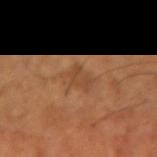This lesion was catalogued during total-body skin photography and was not selected for biopsy.
A 15 mm crop from a total-body photograph taken for skin-cancer surveillance.
The subject is a male about 50 years old.
Approximately 3.5 mm at its widest.
Automated tile analysis of the lesion measured a mean CIELAB color near L≈46 a*≈21 b*≈34, a lesion–skin lightness drop of about 6, and a lesion-to-skin contrast of about 5 (normalized; higher = more distinct). It also reported border irregularity of about 4 on a 0–10 scale and peripheral color asymmetry of about 0.5.
From the left forearm.